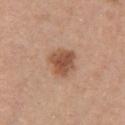Q: What is the anatomic site?
A: the chest
Q: How large is the lesion?
A: ≈3.5 mm
Q: Automated lesion metrics?
A: an area of roughly 9 mm² and a shape-asymmetry score of about 0.2 (0 = symmetric); an average lesion color of about L≈52 a*≈22 b*≈32 (CIELAB) and a normalized lesion–skin contrast near 8.5; a nevus-likeness score of about 95/100 and a detector confidence of about 100 out of 100 that the crop contains a lesion
Q: Who is the patient?
A: female, about 30 years old
Q: How was the tile lit?
A: white-light illumination
Q: What is the imaging modality?
A: total-body-photography crop, ~15 mm field of view